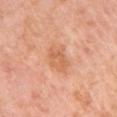biopsy status: no biopsy performed (imaged during a skin exam)
lighting: white-light
patient: female, aged approximately 55
location: the left upper arm
lesion size: ~4 mm (longest diameter)
image source: 15 mm crop, total-body photography
automated metrics: an area of roughly 8.5 mm², an eccentricity of roughly 0.65, and two-axis asymmetry of about 0.2; a border-irregularity index near 2.5/10, a within-lesion color-variation index near 2.5/10, and radial color variation of about 1